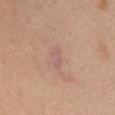– notes — catalogued during a skin exam; not biopsied
– body site — the front of the torso
– image — total-body-photography crop, ~15 mm field of view
– size — about 3 mm
– tile lighting — white-light
– patient — female, in their mid- to late 60s